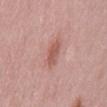Case summary:
• follow-up · total-body-photography surveillance lesion; no biopsy
• lighting · white-light illumination
• TBP lesion metrics · an average lesion color of about L≈56 a*≈25 b*≈26 (CIELAB) and a normalized border contrast of about 7; radial color variation of about 0.5
• body site · the mid back
• patient · female, in their 70s
• diameter · ≈3.5 mm
• image · ~15 mm tile from a whole-body skin photo